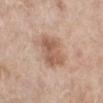Clinical impression: This lesion was catalogued during total-body skin photography and was not selected for biopsy. Image and clinical context: An algorithmic analysis of the crop reported a lesion area of about 15 mm², an outline eccentricity of about 0.5 (0 = round, 1 = elongated), and two-axis asymmetry of about 0.2. The analysis additionally found a lesion color around L≈59 a*≈19 b*≈29 in CIELAB and a normalized border contrast of about 7. The analysis additionally found radial color variation of about 1.5. A female patient in their mid- to late 60s. A close-up tile cropped from a whole-body skin photograph, about 15 mm across. Located on the left lower leg. About 4.5 mm across.